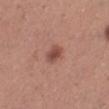workup — total-body-photography surveillance lesion; no biopsy | site — the leg | image — 15 mm crop, total-body photography | lesion diameter — ~2.5 mm (longest diameter) | lighting — white-light illumination | patient — female, aged around 30.